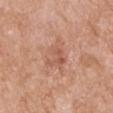<case>
<biopsy_status>not biopsied; imaged during a skin examination</biopsy_status>
<site>left upper arm</site>
<automated_metrics>
  <area_mm2_approx>7.0</area_mm2_approx>
  <shape_asymmetry>0.4</shape_asymmetry>
  <border_irregularity_0_10>4.5</border_irregularity_0_10>
  <color_variation_0_10>3.5</color_variation_0_10>
  <peripheral_color_asymmetry>1.5</peripheral_color_asymmetry>
</automated_metrics>
<lighting>white-light</lighting>
<lesion_size>
  <long_diameter_mm_approx>3.5</long_diameter_mm_approx>
</lesion_size>
<patient>
  <sex>male</sex>
  <age_approx>60</age_approx>
</patient>
<image>
  <source>total-body photography crop</source>
  <field_of_view_mm>15</field_of_view_mm>
</image>
</case>